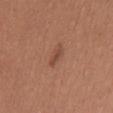notes = no biopsy performed (imaged during a skin exam) | location = the back | illumination = white-light illumination | subject = female, aged 23 to 27 | imaging modality = total-body-photography crop, ~15 mm field of view | lesion diameter = about 2.5 mm.